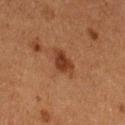follow-up: no biopsy performed (imaged during a skin exam)
lesion diameter: ~3 mm (longest diameter)
image source: ~15 mm crop, total-body skin-cancer survey
anatomic site: the left thigh
TBP lesion metrics: a footprint of about 5 mm² and an eccentricity of roughly 0.7; a lesion color around L≈31 a*≈21 b*≈28 in CIELAB, about 9 CIELAB-L* units darker than the surrounding skin, and a lesion-to-skin contrast of about 9 (normalized; higher = more distinct); a classifier nevus-likeness of about 85/100 and a detector confidence of about 100 out of 100 that the crop contains a lesion
subject: female, approximately 50 years of age